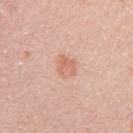Recorded during total-body skin imaging; not selected for excision or biopsy. Located on the arm. The subject is a female approximately 55 years of age. Cropped from a total-body skin-imaging series; the visible field is about 15 mm.